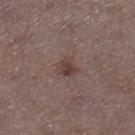{
  "biopsy_status": "not biopsied; imaged during a skin examination",
  "image": {
    "source": "total-body photography crop",
    "field_of_view_mm": 15
  },
  "patient": {
    "sex": "female",
    "age_approx": 40
  },
  "site": "right lower leg",
  "lighting": "white-light",
  "automated_metrics": {
    "area_mm2_approx": 4.5,
    "shape_asymmetry": 0.3,
    "border_irregularity_0_10": 2.5,
    "peripheral_color_asymmetry": 1.0
  }
}